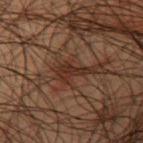| key | value |
|---|---|
| illumination | cross-polarized illumination |
| imaging modality | ~15 mm crop, total-body skin-cancer survey |
| patient | male, approximately 50 years of age |
| site | the front of the torso |